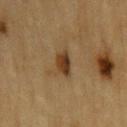biopsy status=no biopsy performed (imaged during a skin exam) | diameter=~2.5 mm (longest diameter) | site=the left upper arm | tile lighting=cross-polarized | image source=total-body-photography crop, ~15 mm field of view | subject=male, in their mid- to late 80s or older.